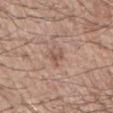workup: catalogued during a skin exam; not biopsied
image source: ~15 mm tile from a whole-body skin photo
location: the right forearm
subject: male, roughly 65 years of age
automated lesion analysis: an area of roughly 3 mm², a shape eccentricity near 0.75, and a shape-asymmetry score of about 0.55 (0 = symmetric); a nevus-likeness score of about 0/100 and lesion-presence confidence of about 100/100
diameter: ~3 mm (longest diameter)